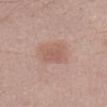Clinical impression: Part of a total-body skin-imaging series; this lesion was reviewed on a skin check and was not flagged for biopsy. Context: A male patient roughly 50 years of age. Imaged with white-light lighting. A close-up tile cropped from a whole-body skin photograph, about 15 mm across. Longest diameter approximately 3.5 mm. The lesion is on the left forearm.